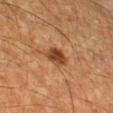Impression:
This lesion was catalogued during total-body skin photography and was not selected for biopsy.
Background:
Approximately 3 mm at its widest. An algorithmic analysis of the crop reported a footprint of about 5.5 mm², an eccentricity of roughly 0.5, and a symmetry-axis asymmetry near 0.25. It also reported a lesion color around L≈40 a*≈23 b*≈33 in CIELAB, a lesion–skin lightness drop of about 12, and a lesion-to-skin contrast of about 10 (normalized; higher = more distinct). Cropped from a total-body skin-imaging series; the visible field is about 15 mm. A male subject aged around 60. The tile uses cross-polarized illumination. Located on the right lower leg.